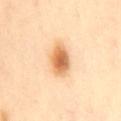workup — total-body-photography surveillance lesion; no biopsy | image source — ~15 mm tile from a whole-body skin photo | site — the mid back | patient — female, aged 43 to 47.